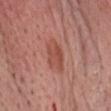lighting: white-light illumination; image: total-body-photography crop, ~15 mm field of view; site: the chest; patient: male, aged approximately 60.